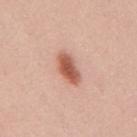This lesion was catalogued during total-body skin photography and was not selected for biopsy. Approximately 4.5 mm at its widest. Located on the mid back. The lesion-visualizer software estimated a footprint of about 7 mm², a shape eccentricity near 0.85, and a symmetry-axis asymmetry near 0.25. Captured under white-light illumination. A female subject, in their mid- to late 20s. A 15 mm crop from a total-body photograph taken for skin-cancer surveillance.